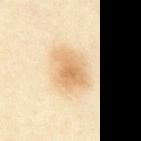Part of a total-body skin-imaging series; this lesion was reviewed on a skin check and was not flagged for biopsy. A male subject aged around 30. A region of skin cropped from a whole-body photographic capture, roughly 15 mm wide. The lesion is on the abdomen. About 4.5 mm across.